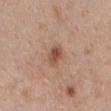The lesion was tiled from a total-body skin photograph and was not biopsied. About 2.5 mm across. The lesion is located on the mid back. This image is a 15 mm lesion crop taken from a total-body photograph. The tile uses white-light illumination. The patient is a female aged 38–42.